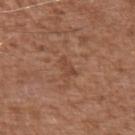Clinical impression: The lesion was photographed on a routine skin check and not biopsied; there is no pathology result. Image and clinical context: Longest diameter approximately 2.5 mm. A close-up tile cropped from a whole-body skin photograph, about 15 mm across. The lesion is located on the left upper arm. The patient is a male in their mid-70s. Captured under white-light illumination. The lesion-visualizer software estimated a lesion–skin lightness drop of about 6. And it measured a border-irregularity rating of about 4/10, a color-variation rating of about 1/10, and a peripheral color-asymmetry measure near 0.